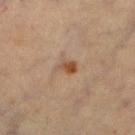{
  "biopsy_status": "not biopsied; imaged during a skin examination"
}